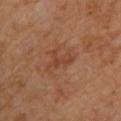Q: Is there a histopathology result?
A: total-body-photography surveillance lesion; no biopsy
Q: How was this image acquired?
A: total-body-photography crop, ~15 mm field of view
Q: Automated lesion metrics?
A: a mean CIELAB color near L≈40 a*≈23 b*≈30 and a normalized lesion–skin contrast near 5.5; a peripheral color-asymmetry measure near 0
Q: Where on the body is the lesion?
A: the upper back
Q: Who is the patient?
A: female, aged around 70
Q: What is the lesion's diameter?
A: ~3 mm (longest diameter)